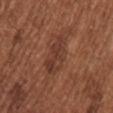Impression:
Recorded during total-body skin imaging; not selected for excision or biopsy.
Clinical summary:
Located on the upper back. The patient is a female roughly 65 years of age. A lesion tile, about 15 mm wide, cut from a 3D total-body photograph.